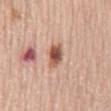Notes:
– biopsy status: catalogued during a skin exam; not biopsied
– site: the mid back
– lesion diameter: ~3 mm (longest diameter)
– patient: female, in their mid- to late 60s
– acquisition: total-body-photography crop, ~15 mm field of view
– lighting: white-light illumination